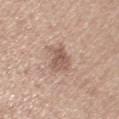biopsy_status: not biopsied; imaged during a skin examination
patient:
  sex: female
  age_approx: 65
automated_metrics:
  area_mm2_approx: 7.5
  eccentricity: 0.65
  shape_asymmetry: 0.25
  cielab_L: 57
  cielab_a: 18
  cielab_b: 25
  vs_skin_darker_L: 10.0
  vs_skin_contrast_norm: 7.0
  border_irregularity_0_10: 3.0
  color_variation_0_10: 3.5
  nevus_likeness_0_100: 15
  lesion_detection_confidence_0_100: 100
image:
  source: total-body photography crop
  field_of_view_mm: 15
site: left upper arm
lesion_size:
  long_diameter_mm_approx: 3.5